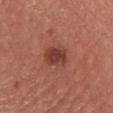biopsy_status: not biopsied; imaged during a skin examination
site: chest
automated_metrics:
  area_mm2_approx: 7.5
  eccentricity: 0.55
  shape_asymmetry: 0.15
  vs_skin_darker_L: 10.0
  border_irregularity_0_10: 1.5
  color_variation_0_10: 3.5
  peripheral_color_asymmetry: 1.0
  lesion_detection_confidence_0_100: 100
lesion_size:
  long_diameter_mm_approx: 3.0
image:
  source: total-body photography crop
  field_of_view_mm: 15
lighting: white-light
patient:
  sex: female
  age_approx: 65